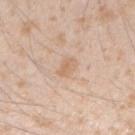Impression:
Recorded during total-body skin imaging; not selected for excision or biopsy.
Image and clinical context:
The lesion is located on the right forearm. Measured at roughly 2.5 mm in maximum diameter. This image is a 15 mm lesion crop taken from a total-body photograph. The patient is a male in their mid- to late 20s.